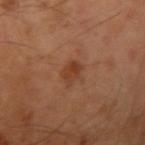Findings:
- workup: no biopsy performed (imaged during a skin exam)
- imaging modality: 15 mm crop, total-body photography
- patient: male, aged around 50
- site: the left arm
- lesion size: about 2.5 mm
- automated metrics: a footprint of about 4.5 mm², an outline eccentricity of about 0.65 (0 = round, 1 = elongated), and a symmetry-axis asymmetry near 0.2; an automated nevus-likeness rating near 40 out of 100 and a lesion-detection confidence of about 100/100
- tile lighting: cross-polarized